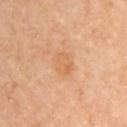<record>
  <biopsy_status>not biopsied; imaged during a skin examination</biopsy_status>
  <site>upper back</site>
  <image>
    <source>total-body photography crop</source>
    <field_of_view_mm>15</field_of_view_mm>
  </image>
  <lighting>cross-polarized</lighting>
  <patient>
    <sex>female</sex>
    <age_approx>50</age_approx>
  </patient>
</record>